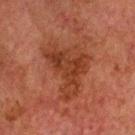workup — imaged on a skin check; not biopsied | lighting — cross-polarized illumination | subject — male, roughly 60 years of age | image — 15 mm crop, total-body photography | location — the head or neck | lesion size — ~7.5 mm (longest diameter) | image-analysis metrics — a lesion area of about 21 mm², an eccentricity of roughly 0.8, and a symmetry-axis asymmetry near 0.6; an average lesion color of about L≈29 a*≈22 b*≈27 (CIELAB), a lesion–skin lightness drop of about 7, and a normalized border contrast of about 7; border irregularity of about 7.5 on a 0–10 scale and internal color variation of about 4 on a 0–10 scale.